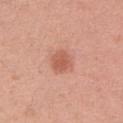| key | value |
|---|---|
| notes | catalogued during a skin exam; not biopsied |
| acquisition | 15 mm crop, total-body photography |
| site | the right upper arm |
| diameter | ~3 mm (longest diameter) |
| TBP lesion metrics | a footprint of about 5.5 mm², a shape eccentricity near 0.5, and a symmetry-axis asymmetry near 0.15; a lesion color around L≈58 a*≈27 b*≈32 in CIELAB; a border-irregularity index near 1.5/10, internal color variation of about 2 on a 0–10 scale, and a peripheral color-asymmetry measure near 0.5; lesion-presence confidence of about 100/100 |
| subject | female, aged 33 to 37 |